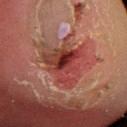notes: imaged on a skin check; not biopsied | size: ~6 mm (longest diameter) | lighting: cross-polarized | image: ~15 mm crop, total-body skin-cancer survey | location: the right lower leg | patient: female, about 50 years old.